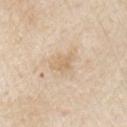| feature | finding |
|---|---|
| follow-up | imaged on a skin check; not biopsied |
| patient | male, approximately 80 years of age |
| lesion size | ~3 mm (longest diameter) |
| location | the right upper arm |
| image source | ~15 mm crop, total-body skin-cancer survey |
| image-analysis metrics | an automated nevus-likeness rating near 0 out of 100 and a detector confidence of about 100 out of 100 that the crop contains a lesion |